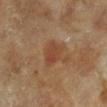Impression: The lesion was tiled from a total-body skin photograph and was not biopsied. Context: A region of skin cropped from a whole-body photographic capture, roughly 15 mm wide. A male subject, approximately 65 years of age. Imaged with cross-polarized lighting. The lesion is located on the left lower leg. An algorithmic analysis of the crop reported a border-irregularity index near 2.5/10 and a peripheral color-asymmetry measure near 1.5. And it measured an automated nevus-likeness rating near 60 out of 100. Measured at roughly 3.5 mm in maximum diameter.